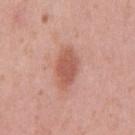| field | value |
|---|---|
| follow-up | no biopsy performed (imaged during a skin exam) |
| illumination | white-light |
| TBP lesion metrics | a lesion area of about 9 mm², an eccentricity of roughly 0.85, and a shape-asymmetry score of about 0.2 (0 = symmetric) |
| diameter | ≈5 mm |
| acquisition | ~15 mm tile from a whole-body skin photo |
| subject | male, in their mid- to late 50s |
| site | the abdomen |